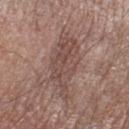The lesion was photographed on a routine skin check and not biopsied; there is no pathology result.
An algorithmic analysis of the crop reported an area of roughly 20 mm² and two-axis asymmetry of about 0.35. The software also gave an average lesion color of about L≈48 a*≈18 b*≈22 (CIELAB) and a normalized lesion–skin contrast near 6.
A 15 mm crop from a total-body photograph taken for skin-cancer surveillance.
This is a white-light tile.
The subject is a male aged 83 or over.
The lesion is on the leg.
About 7.5 mm across.